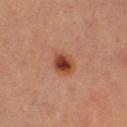{"biopsy_status": "not biopsied; imaged during a skin examination", "lesion_size": {"long_diameter_mm_approx": 2.5}, "image": {"source": "total-body photography crop", "field_of_view_mm": 15}, "patient": {"sex": "female", "age_approx": 35}, "site": "right thigh", "automated_metrics": {"eccentricity": 0.4, "border_irregularity_0_10": 1.5, "color_variation_0_10": 5.0, "peripheral_color_asymmetry": 1.5}}